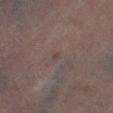Imaged during a routine full-body skin examination; the lesion was not biopsied and no histopathology is available. A female subject, about 80 years old. A 15 mm crop from a total-body photograph taken for skin-cancer surveillance. The lesion is located on the left leg.